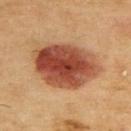Notes:
- follow-up · no biopsy performed (imaged during a skin exam)
- site · the back
- image · 15 mm crop, total-body photography
- patient · male, aged 68–72
- lighting · cross-polarized illumination
- lesion diameter · about 8.5 mm